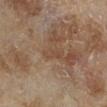workup: catalogued during a skin exam; not biopsied | size: ~3 mm (longest diameter) | subject: female, about 60 years old | image: ~15 mm crop, total-body skin-cancer survey | site: the leg | TBP lesion metrics: a footprint of about 3.5 mm² and a shape-asymmetry score of about 0.3 (0 = symmetric); roughly 5 lightness units darker than nearby skin and a lesion-to-skin contrast of about 5 (normalized; higher = more distinct); a nevus-likeness score of about 0/100 and a detector confidence of about 60 out of 100 that the crop contains a lesion | lighting: cross-polarized.